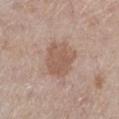No biopsy was performed on this lesion — it was imaged during a full skin examination and was not determined to be concerning. A male subject, aged around 70. The recorded lesion diameter is about 4.5 mm. The lesion is on the left lower leg. A lesion tile, about 15 mm wide, cut from a 3D total-body photograph. Imaged with white-light lighting.